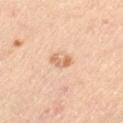Part of a total-body skin-imaging series; this lesion was reviewed on a skin check and was not flagged for biopsy. Imaged with cross-polarized lighting. A female patient roughly 45 years of age. The lesion's longest dimension is about 2.5 mm. A lesion tile, about 15 mm wide, cut from a 3D total-body photograph. On the left thigh. The lesion-visualizer software estimated an area of roughly 3.5 mm², a shape eccentricity near 0.8, and a shape-asymmetry score of about 0.45 (0 = symmetric). And it measured a lesion color around L≈65 a*≈20 b*≈34 in CIELAB, a lesion–skin lightness drop of about 10, and a normalized lesion–skin contrast near 6.5. It also reported a lesion-detection confidence of about 100/100.